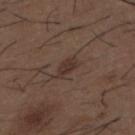A lesion tile, about 15 mm wide, cut from a 3D total-body photograph. Measured at roughly 3 mm in maximum diameter. A male patient, aged 48–52. From the mid back. Captured under white-light illumination. The lesion-visualizer software estimated a footprint of about 4 mm², an eccentricity of roughly 0.85, and a shape-asymmetry score of about 0.25 (0 = symmetric). It also reported an average lesion color of about L≈33 a*≈15 b*≈21 (CIELAB) and roughly 7 lightness units darker than nearby skin. The software also gave a border-irregularity index near 3/10, internal color variation of about 2 on a 0–10 scale, and a peripheral color-asymmetry measure near 0.5.